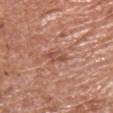follow-up — no biopsy performed (imaged during a skin exam) | illumination — white-light | imaging modality — ~15 mm crop, total-body skin-cancer survey | subject — male, in their mid-60s | location — the chest.